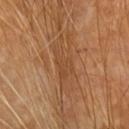Q: Was this lesion biopsied?
A: total-body-photography surveillance lesion; no biopsy
Q: What are the patient's age and sex?
A: male, roughly 60 years of age
Q: How was this image acquired?
A: 15 mm crop, total-body photography
Q: Lesion size?
A: ≈6.5 mm
Q: What is the anatomic site?
A: the arm
Q: What lighting was used for the tile?
A: cross-polarized illumination